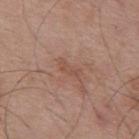Longest diameter approximately 3 mm.
A lesion tile, about 15 mm wide, cut from a 3D total-body photograph.
The subject is a male approximately 55 years of age.
The lesion is located on the mid back.